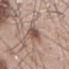This lesion was catalogued during total-body skin photography and was not selected for biopsy. A 15 mm crop from a total-body photograph taken for skin-cancer surveillance. An algorithmic analysis of the crop reported an area of roughly 7.5 mm², an outline eccentricity of about 0.95 (0 = round, 1 = elongated), and a shape-asymmetry score of about 0.2 (0 = symmetric). And it measured an automated nevus-likeness rating near 0 out of 100 and a lesion-detection confidence of about 65/100. A male patient, in their mid-50s. Located on the mid back. Captured under white-light illumination. About 5.5 mm across.